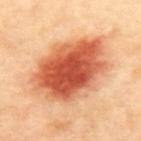Part of a total-body skin-imaging series; this lesion was reviewed on a skin check and was not flagged for biopsy.